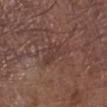follow-up: imaged on a skin check; not biopsied | lesion diameter: ~4.5 mm (longest diameter) | subject: male, aged approximately 55 | lighting: white-light illumination | anatomic site: the left lower leg | automated lesion analysis: a within-lesion color-variation index near 2/10 | image source: ~15 mm tile from a whole-body skin photo.